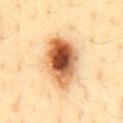{"biopsy_status": "not biopsied; imaged during a skin examination", "lesion_size": {"long_diameter_mm_approx": 6.5}, "lighting": "cross-polarized", "image": {"source": "total-body photography crop", "field_of_view_mm": 15}, "site": "front of the torso", "patient": {"sex": "male", "age_approx": 45}}